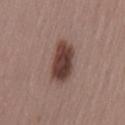Impression: Part of a total-body skin-imaging series; this lesion was reviewed on a skin check and was not flagged for biopsy. Acquisition and patient details: Measured at roughly 5 mm in maximum diameter. The subject is a female roughly 45 years of age. A region of skin cropped from a whole-body photographic capture, roughly 15 mm wide. Located on the left thigh.